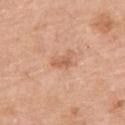  biopsy_status: not biopsied; imaged during a skin examination
  image:
    source: total-body photography crop
    field_of_view_mm: 15
  patient:
    sex: female
    age_approx: 65
  site: right upper arm
  lighting: white-light
  automated_metrics:
    area_mm2_approx: 3.0
    eccentricity: 0.9
    shape_asymmetry: 0.3
    border_irregularity_0_10: 3.0
    color_variation_0_10: 1.0
    peripheral_color_asymmetry: 0.0
  lesion_size:
    long_diameter_mm_approx: 3.0